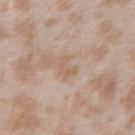The lesion was photographed on a routine skin check and not biopsied; there is no pathology result.
The recorded lesion diameter is about 2.5 mm.
A female subject in their mid-20s.
Imaged with white-light lighting.
The lesion-visualizer software estimated a lesion area of about 2.5 mm², an outline eccentricity of about 0.85 (0 = round, 1 = elongated), and two-axis asymmetry of about 0.65. The analysis additionally found a border-irregularity index near 8/10, a within-lesion color-variation index near 0/10, and radial color variation of about 0. And it measured a classifier nevus-likeness of about 0/100 and a detector confidence of about 100 out of 100 that the crop contains a lesion.
Located on the left forearm.
A close-up tile cropped from a whole-body skin photograph, about 15 mm across.